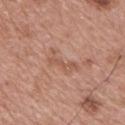{
  "biopsy_status": "not biopsied; imaged during a skin examination",
  "site": "upper back",
  "image": {
    "source": "total-body photography crop",
    "field_of_view_mm": 15
  },
  "lesion_size": {
    "long_diameter_mm_approx": 4.5
  },
  "patient": {
    "sex": "male",
    "age_approx": 65
  },
  "automated_metrics": {
    "cielab_L": 55,
    "cielab_a": 22,
    "cielab_b": 29,
    "vs_skin_darker_L": 7.0
  }
}